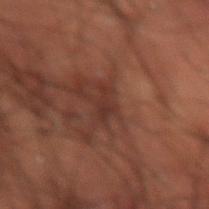workup: total-body-photography surveillance lesion; no biopsy | acquisition: 15 mm crop, total-body photography | subject: male, roughly 50 years of age | size: ≈3 mm | site: the lower back | image-analysis metrics: a lesion area of about 3 mm², a shape eccentricity near 0.85, and a shape-asymmetry score of about 0.4 (0 = symmetric); a border-irregularity rating of about 5/10, a color-variation rating of about 1/10, and radial color variation of about 0.5 | tile lighting: cross-polarized illumination.